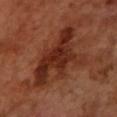tile lighting=cross-polarized; TBP lesion metrics=a mean CIELAB color near L≈28 a*≈25 b*≈28 and a normalized border contrast of about 9.5; acquisition=~15 mm tile from a whole-body skin photo; subject=female, in their 70s; site=the arm.